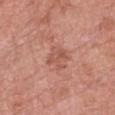<case>
  <biopsy_status>not biopsied; imaged during a skin examination</biopsy_status>
  <lesion_size>
    <long_diameter_mm_approx>3.0</long_diameter_mm_approx>
  </lesion_size>
  <automated_metrics>
    <area_mm2_approx>6.0</area_mm2_approx>
    <eccentricity>0.4</eccentricity>
    <shape_asymmetry>0.3</shape_asymmetry>
    <lesion_detection_confidence_0_100>100</lesion_detection_confidence_0_100>
  </automated_metrics>
  <patient>
    <sex>female</sex>
    <age_approx>70</age_approx>
  </patient>
  <site>chest</site>
  <image>
    <source>total-body photography crop</source>
    <field_of_view_mm>15</field_of_view_mm>
  </image>
</case>